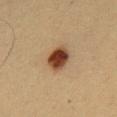This lesion was catalogued during total-body skin photography and was not selected for biopsy.
A close-up tile cropped from a whole-body skin photograph, about 15 mm across.
Imaged with cross-polarized lighting.
A male subject roughly 35 years of age.
The lesion is located on the chest.
Approximately 3.5 mm at its widest.